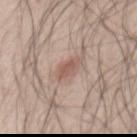* biopsy status: imaged on a skin check; not biopsied
* lesion diameter: about 3.5 mm
* site: the front of the torso
* imaging modality: total-body-photography crop, ~15 mm field of view
* subject: male, roughly 25 years of age